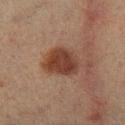Impression: Part of a total-body skin-imaging series; this lesion was reviewed on a skin check and was not flagged for biopsy. Clinical summary: A close-up tile cropped from a whole-body skin photograph, about 15 mm across. Located on the left lower leg. A female subject, in their mid-50s.